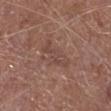- follow-up — imaged on a skin check; not biopsied
- image-analysis metrics — a symmetry-axis asymmetry near 0.35; a nevus-likeness score of about 0/100 and a detector confidence of about 75 out of 100 that the crop contains a lesion
- acquisition — 15 mm crop, total-body photography
- site — the right lower leg
- diameter — ≈5 mm
- subject — male, roughly 75 years of age